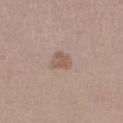This lesion was catalogued during total-body skin photography and was not selected for biopsy. A female patient about 20 years old. About 2.5 mm across. A lesion tile, about 15 mm wide, cut from a 3D total-body photograph. On the right lower leg.